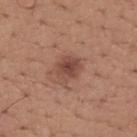workup: no biopsy performed (imaged during a skin exam)
diameter: ≈3 mm
body site: the mid back
lighting: white-light
subject: male, in their mid- to late 60s
image source: 15 mm crop, total-body photography